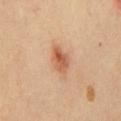The tile uses cross-polarized illumination. On the chest. A 15 mm close-up extracted from a 3D total-body photography capture. A male subject, aged 53 to 57.